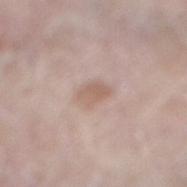Assessment: No biopsy was performed on this lesion — it was imaged during a full skin examination and was not determined to be concerning. Image and clinical context: A male subject roughly 80 years of age. An algorithmic analysis of the crop reported a border-irregularity index near 2/10, a color-variation rating of about 2.5/10, and radial color variation of about 1. Measured at roughly 3 mm in maximum diameter. This image is a 15 mm lesion crop taken from a total-body photograph. On the right lower leg.